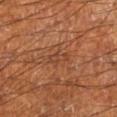Findings:
* follow-up: total-body-photography surveillance lesion; no biopsy
* tile lighting: cross-polarized illumination
* image source: ~15 mm crop, total-body skin-cancer survey
* location: the left lower leg
* patient: male, aged approximately 65
* size: ≈2.5 mm